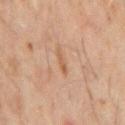Q: Was a biopsy performed?
A: imaged on a skin check; not biopsied
Q: What is the anatomic site?
A: the mid back
Q: Lesion size?
A: ~3 mm (longest diameter)
Q: What did automated image analysis measure?
A: a shape eccentricity near 0.95 and a symmetry-axis asymmetry near 0.35; a lesion color around L≈54 a*≈20 b*≈32 in CIELAB, a lesion–skin lightness drop of about 7, and a normalized lesion–skin contrast near 5.5
Q: What are the patient's age and sex?
A: male, aged 58 to 62
Q: Illumination type?
A: cross-polarized illumination
Q: How was this image acquired?
A: 15 mm crop, total-body photography